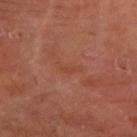Imaged during a routine full-body skin examination; the lesion was not biopsied and no histopathology is available. This is a cross-polarized tile. The patient is roughly 65 years of age. A 15 mm close-up extracted from a 3D total-body photography capture. Longest diameter approximately 3 mm. The lesion is located on the right forearm. Automated image analysis of the tile measured an automated nevus-likeness rating near 0 out of 100 and a lesion-detection confidence of about 95/100.